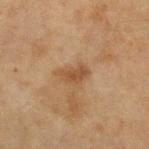biopsy_status: not biopsied; imaged during a skin examination
automated_metrics:
  eccentricity: 0.85
  shape_asymmetry: 0.35
  border_irregularity_0_10: 3.5
  color_variation_0_10: 2.0
  peripheral_color_asymmetry: 0.5
  lesion_detection_confidence_0_100: 100
image:
  source: total-body photography crop
  field_of_view_mm: 15
site: arm
lighting: cross-polarized
patient:
  sex: female
  age_approx: 40